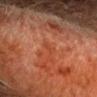Q: Patient demographics?
A: male, approximately 60 years of age
Q: How was this image acquired?
A: ~15 mm tile from a whole-body skin photo
Q: What is the anatomic site?
A: the head or neck